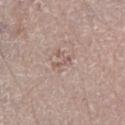Q: Was a biopsy performed?
A: imaged on a skin check; not biopsied